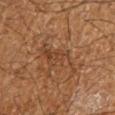<lesion>
  <biopsy_status>not biopsied; imaged during a skin examination</biopsy_status>
  <image>
    <source>total-body photography crop</source>
    <field_of_view_mm>15</field_of_view_mm>
  </image>
  <site>left upper arm</site>
  <patient>
    <sex>male</sex>
    <age_approx>60</age_approx>
  </patient>
</lesion>